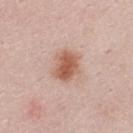Notes:
* biopsy status: catalogued during a skin exam; not biopsied
* automated metrics: a lesion color around L≈58 a*≈22 b*≈30 in CIELAB, roughly 12 lightness units darker than nearby skin, and a lesion-to-skin contrast of about 9 (normalized; higher = more distinct); a peripheral color-asymmetry measure near 1; a classifier nevus-likeness of about 100/100 and lesion-presence confidence of about 100/100
* image: total-body-photography crop, ~15 mm field of view
* size: ≈4 mm
* patient: male, roughly 25 years of age
* illumination: white-light illumination
* site: the upper back